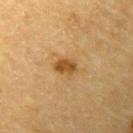Image and clinical context: A region of skin cropped from a whole-body photographic capture, roughly 15 mm wide. Imaged with cross-polarized lighting. The lesion-visualizer software estimated an outline eccentricity of about 0.7 (0 = round, 1 = elongated). A male patient aged 83 or over. The lesion is on the left upper arm.